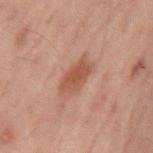The lesion was photographed on a routine skin check and not biopsied; there is no pathology result. A 15 mm close-up extracted from a 3D total-body photography capture. Captured under cross-polarized illumination. Automated image analysis of the tile measured a footprint of about 7.5 mm² and an outline eccentricity of about 0.9 (0 = round, 1 = elongated). The software also gave a color-variation rating of about 2.5/10 and a peripheral color-asymmetry measure near 0.5. The analysis additionally found an automated nevus-likeness rating near 50 out of 100. Longest diameter approximately 4.5 mm. The lesion is on the mid back. A male subject, aged 58 to 62.